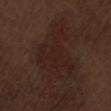Q: Is there a histopathology result?
A: no biopsy performed (imaged during a skin exam)
Q: Who is the patient?
A: male, aged 68 to 72
Q: Lesion location?
A: the lower back
Q: What kind of image is this?
A: total-body-photography crop, ~15 mm field of view